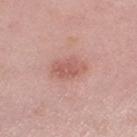Q: Was this lesion biopsied?
A: catalogued during a skin exam; not biopsied
Q: What did automated image analysis measure?
A: a mean CIELAB color near L≈58 a*≈25 b*≈26, about 9 CIELAB-L* units darker than the surrounding skin, and a lesion-to-skin contrast of about 6 (normalized; higher = more distinct)
Q: What kind of image is this?
A: ~15 mm crop, total-body skin-cancer survey
Q: Who is the patient?
A: female, roughly 40 years of age
Q: What is the lesion's diameter?
A: ≈4 mm
Q: Where on the body is the lesion?
A: the right thigh